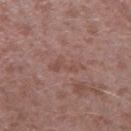biopsy status — catalogued during a skin exam; not biopsied
lesion diameter — ~4 mm (longest diameter)
TBP lesion metrics — a footprint of about 5 mm² and a shape-asymmetry score of about 0.55 (0 = symmetric); about 6 CIELAB-L* units darker than the surrounding skin and a lesion-to-skin contrast of about 5 (normalized; higher = more distinct); an automated nevus-likeness rating near 0 out of 100 and lesion-presence confidence of about 95/100
acquisition — ~15 mm crop, total-body skin-cancer survey
patient — male, in their mid- to late 40s
location — the left thigh